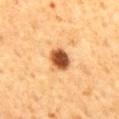follow-up — catalogued during a skin exam; not biopsied
subject — male, in their 60s
image source — total-body-photography crop, ~15 mm field of view
lesion diameter — about 3 mm
site — the mid back
lighting — cross-polarized illumination